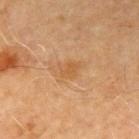notes = no biopsy performed (imaged during a skin exam) | diameter = ≈2.5 mm | location = the left upper arm | image = total-body-photography crop, ~15 mm field of view | patient = male, in their 70s.